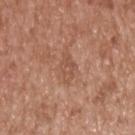{
  "biopsy_status": "not biopsied; imaged during a skin examination",
  "patient": {
    "sex": "male",
    "age_approx": 65
  },
  "image": {
    "source": "total-body photography crop",
    "field_of_view_mm": 15
  },
  "site": "upper back",
  "automated_metrics": {
    "area_mm2_approx": 4.0,
    "color_variation_0_10": 2.5,
    "peripheral_color_asymmetry": 1.0,
    "nevus_likeness_0_100": 0,
    "lesion_detection_confidence_0_100": 100
  },
  "lesion_size": {
    "long_diameter_mm_approx": 3.5
  }
}